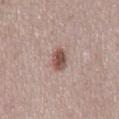No biopsy was performed on this lesion — it was imaged during a full skin examination and was not determined to be concerning. The tile uses white-light illumination. A 15 mm close-up tile from a total-body photography series done for melanoma screening. From the mid back. The lesion's longest dimension is about 3 mm. Automated image analysis of the tile measured a lesion area of about 5 mm², an eccentricity of roughly 0.8, and a shape-asymmetry score of about 0.2 (0 = symmetric). And it measured a border-irregularity index near 2.5/10, a color-variation rating of about 4/10, and radial color variation of about 1.5. The subject is a male in their 70s.